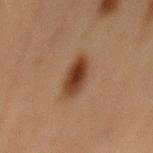Clinical impression:
The lesion was tiled from a total-body skin photograph and was not biopsied.
Image and clinical context:
A 15 mm crop from a total-body photograph taken for skin-cancer surveillance. The lesion is on the abdomen. The patient is a male aged 68 to 72.